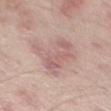Assessment: This lesion was catalogued during total-body skin photography and was not selected for biopsy. Context: On the left thigh. The subject is a male aged 63–67. A roughly 15 mm field-of-view crop from a total-body skin photograph.